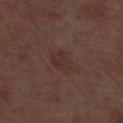| field | value |
|---|---|
| follow-up | no biopsy performed (imaged during a skin exam) |
| TBP lesion metrics | a mean CIELAB color near L≈31 a*≈16 b*≈20 and a normalized border contrast of about 5; a border-irregularity index near 2.5/10, a within-lesion color-variation index near 2/10, and a peripheral color-asymmetry measure near 0.5 |
| image | ~15 mm crop, total-body skin-cancer survey |
| subject | male, in their 50s |